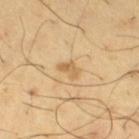notes=imaged on a skin check; not biopsied
site=the right thigh
imaging modality=15 mm crop, total-body photography
subject=male, aged 63 to 67
lighting=cross-polarized illumination